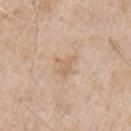Captured during whole-body skin photography for melanoma surveillance; the lesion was not biopsied. A region of skin cropped from a whole-body photographic capture, roughly 15 mm wide. A male patient, aged around 65. The lesion is on the left upper arm. The tile uses white-light illumination.